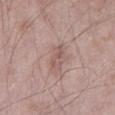Findings:
• workup: imaged on a skin check; not biopsied
• automated lesion analysis: an area of roughly 3.5 mm² and two-axis asymmetry of about 0.3; a mean CIELAB color near L≈55 a*≈19 b*≈22, a lesion–skin lightness drop of about 8, and a normalized lesion–skin contrast near 6; a border-irregularity rating of about 4/10 and a peripheral color-asymmetry measure near 0.5; a classifier nevus-likeness of about 0/100
• image source: 15 mm crop, total-body photography
• patient: male, in their 50s
• lesion diameter: ≈3.5 mm
• anatomic site: the left thigh
• tile lighting: white-light